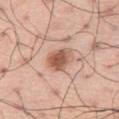Impression: Recorded during total-body skin imaging; not selected for excision or biopsy. Image and clinical context: A region of skin cropped from a whole-body photographic capture, roughly 15 mm wide. A male patient, aged 68 to 72. Located on the abdomen.